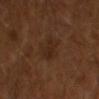Q: Illumination type?
A: cross-polarized
Q: Patient demographics?
A: male, aged around 65
Q: How large is the lesion?
A: ~3 mm (longest diameter)
Q: What is the imaging modality?
A: ~15 mm tile from a whole-body skin photo